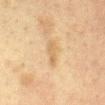A female patient in their 40s. The lesion-visualizer software estimated a border-irregularity index near 4/10, internal color variation of about 1 on a 0–10 scale, and radial color variation of about 0.5. It also reported an automated nevus-likeness rating near 0 out of 100 and a lesion-detection confidence of about 100/100. Captured under cross-polarized illumination. A roughly 15 mm field-of-view crop from a total-body skin photograph. From the chest.